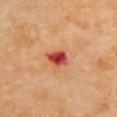A 15 mm close-up tile from a total-body photography series done for melanoma screening.
The subject is a female roughly 55 years of age.
This is a cross-polarized tile.
The lesion is on the chest.